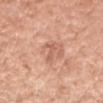workup: catalogued during a skin exam; not biopsied
acquisition: ~15 mm crop, total-body skin-cancer survey
tile lighting: white-light illumination
patient: female, in their mid-50s
image-analysis metrics: a lesion area of about 3 mm², an eccentricity of roughly 0.95, and a symmetry-axis asymmetry near 0.4; a lesion color around L≈60 a*≈24 b*≈31 in CIELAB, about 8 CIELAB-L* units darker than the surrounding skin, and a lesion-to-skin contrast of about 5 (normalized; higher = more distinct)
size: ≈3 mm
body site: the right forearm